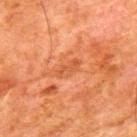Case summary:
- site — the upper back
- lesion size — ~3 mm (longest diameter)
- image — 15 mm crop, total-body photography
- patient — male, aged around 80
- illumination — cross-polarized illumination